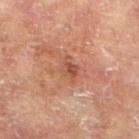A 15 mm close-up extracted from a 3D total-body photography capture. A female patient, aged 73 to 77. The lesion is on the leg.